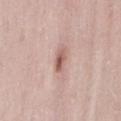Clinical impression: This lesion was catalogued during total-body skin photography and was not selected for biopsy. Background: A region of skin cropped from a whole-body photographic capture, roughly 15 mm wide. From the lower back. Approximately 2.5 mm at its widest. The patient is a female aged approximately 30. An algorithmic analysis of the crop reported a classifier nevus-likeness of about 80/100 and lesion-presence confidence of about 100/100. The tile uses white-light illumination.